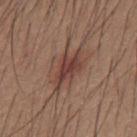The lesion was photographed on a routine skin check and not biopsied; there is no pathology result.
The patient is a male in their 20s.
Approximately 5 mm at its widest.
Cropped from a total-body skin-imaging series; the visible field is about 15 mm.
The lesion is on the mid back.